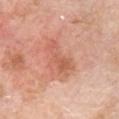Assessment: The lesion was photographed on a routine skin check and not biopsied; there is no pathology result. Acquisition and patient details: The lesion is on the chest. Imaged with white-light lighting. A lesion tile, about 15 mm wide, cut from a 3D total-body photograph. The subject is a male in their 60s. About 5 mm across.